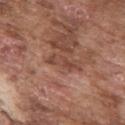workup=total-body-photography surveillance lesion; no biopsy | subject=male, roughly 75 years of age | tile lighting=white-light illumination | size=about 3.5 mm | image source=total-body-photography crop, ~15 mm field of view | TBP lesion metrics=a symmetry-axis asymmetry near 0.35; a border-irregularity rating of about 4/10 and a within-lesion color-variation index near 4/10; a nevus-likeness score of about 0/100 and a detector confidence of about 100 out of 100 that the crop contains a lesion | body site=the right upper arm.